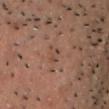Q: Was a biopsy performed?
A: no biopsy performed (imaged during a skin exam)
Q: How large is the lesion?
A: ~2.5 mm (longest diameter)
Q: What is the imaging modality?
A: 15 mm crop, total-body photography
Q: Who is the patient?
A: male, approximately 55 years of age
Q: How was the tile lit?
A: cross-polarized illumination
Q: What is the anatomic site?
A: the head or neck
Q: Automated lesion metrics?
A: a lesion area of about 3 mm², an outline eccentricity of about 0.8 (0 = round, 1 = elongated), and a shape-asymmetry score of about 0.45 (0 = symmetric); an average lesion color of about L≈44 a*≈18 b*≈26 (CIELAB), about 6 CIELAB-L* units darker than the surrounding skin, and a lesion-to-skin contrast of about 4.5 (normalized; higher = more distinct); a border-irregularity rating of about 5/10 and a peripheral color-asymmetry measure near 0